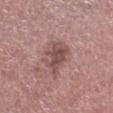{"biopsy_status": "not biopsied; imaged during a skin examination", "lighting": "white-light", "image": {"source": "total-body photography crop", "field_of_view_mm": 15}, "patient": {"sex": "male", "age_approx": 75}, "automated_metrics": {"area_mm2_approx": 9.5, "shape_asymmetry": 0.3, "cielab_L": 49, "cielab_a": 19, "cielab_b": 20, "vs_skin_darker_L": 10.0, "vs_skin_contrast_norm": 7.5, "border_irregularity_0_10": 4.5, "color_variation_0_10": 3.0, "peripheral_color_asymmetry": 1.0}, "site": "right lower leg"}